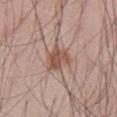Q: Was a biopsy performed?
A: no biopsy performed (imaged during a skin exam)
Q: What are the patient's age and sex?
A: male, aged approximately 55
Q: Lesion location?
A: the abdomen
Q: Lesion size?
A: about 3 mm
Q: How was this image acquired?
A: ~15 mm crop, total-body skin-cancer survey